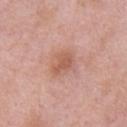The lesion was photographed on a routine skin check and not biopsied; there is no pathology result.
A male subject, aged around 55.
The total-body-photography lesion software estimated border irregularity of about 2.5 on a 0–10 scale, internal color variation of about 3.5 on a 0–10 scale, and a peripheral color-asymmetry measure near 1.
Cropped from a whole-body photographic skin survey; the tile spans about 15 mm.
The lesion is located on the chest.
Longest diameter approximately 3.5 mm.